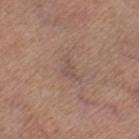Captured during whole-body skin photography for melanoma surveillance; the lesion was not biopsied.
The lesion is located on the leg.
Automated image analysis of the tile measured an average lesion color of about L≈51 a*≈18 b*≈22 (CIELAB). It also reported a border-irregularity index near 6/10, a color-variation rating of about 1/10, and peripheral color asymmetry of about 0.5.
Measured at roughly 3 mm in maximum diameter.
A female subject, about 55 years old.
Captured under white-light illumination.
Cropped from a whole-body photographic skin survey; the tile spans about 15 mm.